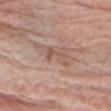biopsy status: no biopsy performed (imaged during a skin exam) | image source: ~15 mm crop, total-body skin-cancer survey | anatomic site: the right upper arm | tile lighting: white-light illumination | subject: male, roughly 80 years of age.